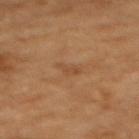Acquisition and patient details: Imaged with cross-polarized lighting. The total-body-photography lesion software estimated a footprint of about 3 mm², an outline eccentricity of about 0.8 (0 = round, 1 = elongated), and two-axis asymmetry of about 0.4. And it measured a classifier nevus-likeness of about 0/100 and a lesion-detection confidence of about 100/100. The lesion's longest dimension is about 2.5 mm. A 15 mm close-up tile from a total-body photography series done for melanoma screening. The patient is a female aged 53 to 57. Located on the upper back.